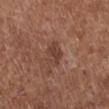The patient is a male in their mid- to late 70s.
Longest diameter approximately 3 mm.
A roughly 15 mm field-of-view crop from a total-body skin photograph.
An algorithmic analysis of the crop reported a lesion color around L≈41 a*≈21 b*≈26 in CIELAB, about 8 CIELAB-L* units darker than the surrounding skin, and a normalized lesion–skin contrast near 6.5. The software also gave a border-irregularity rating of about 3/10 and a peripheral color-asymmetry measure near 1.
Located on the right lower leg.
Captured under white-light illumination.